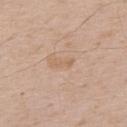Q: Was this lesion biopsied?
A: catalogued during a skin exam; not biopsied
Q: Automated lesion metrics?
A: an area of roughly 2 mm², an eccentricity of roughly 0.95, and two-axis asymmetry of about 0.4
Q: Patient demographics?
A: male, roughly 75 years of age
Q: Lesion size?
A: ≈2.5 mm
Q: Lesion location?
A: the upper back
Q: How was the tile lit?
A: white-light illumination
Q: What is the imaging modality?
A: 15 mm crop, total-body photography